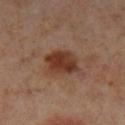No biopsy was performed on this lesion — it was imaged during a full skin examination and was not determined to be concerning. The tile uses cross-polarized illumination. The subject is a female aged approximately 55. The total-body-photography lesion software estimated a footprint of about 10 mm², a shape eccentricity near 0.65, and two-axis asymmetry of about 0.2. The recorded lesion diameter is about 4 mm. A 15 mm crop from a total-body photograph taken for skin-cancer surveillance. The lesion is on the left lower leg.